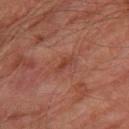<tbp_lesion>
<image>
  <source>total-body photography crop</source>
  <field_of_view_mm>15</field_of_view_mm>
</image>
<site>leg</site>
<patient>
  <sex>male</sex>
  <age_approx>75</age_approx>
</patient>
</tbp_lesion>